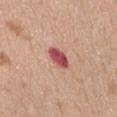Impression:
Recorded during total-body skin imaging; not selected for excision or biopsy.